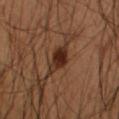Case summary:
* acquisition — 15 mm crop, total-body photography
* site — the right forearm
* lesion diameter — ~3.5 mm (longest diameter)
* patient — male, about 55 years old